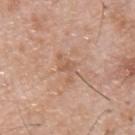{
  "biopsy_status": "not biopsied; imaged during a skin examination",
  "image": {
    "source": "total-body photography crop",
    "field_of_view_mm": 15
  },
  "lesion_size": {
    "long_diameter_mm_approx": 3.5
  },
  "patient": {
    "sex": "male",
    "age_approx": 55
  },
  "automated_metrics": {
    "cielab_L": 59,
    "cielab_a": 20,
    "cielab_b": 31,
    "vs_skin_contrast_norm": 5.0,
    "nevus_likeness_0_100": 0,
    "lesion_detection_confidence_0_100": 100
  },
  "lighting": "white-light"
}